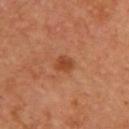No biopsy was performed on this lesion — it was imaged during a full skin examination and was not determined to be concerning.
Captured under cross-polarized illumination.
The patient is a male in their mid- to late 60s.
From the upper back.
Cropped from a total-body skin-imaging series; the visible field is about 15 mm.